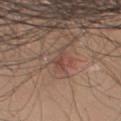A male patient aged around 45. The tile uses white-light illumination. Located on the right upper arm. The lesion's longest dimension is about 2.5 mm. Cropped from a total-body skin-imaging series; the visible field is about 15 mm. An algorithmic analysis of the crop reported border irregularity of about 5.5 on a 0–10 scale, a color-variation rating of about 1.5/10, and peripheral color asymmetry of about 0.5.